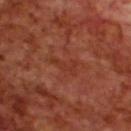Impression: No biopsy was performed on this lesion — it was imaged during a full skin examination and was not determined to be concerning. Image and clinical context: A male subject, about 70 years old. Approximately 3.5 mm at its widest. A 15 mm close-up tile from a total-body photography series done for melanoma screening. The total-body-photography lesion software estimated a lesion area of about 3.5 mm², an outline eccentricity of about 0.9 (0 = round, 1 = elongated), and a symmetry-axis asymmetry near 0.55. The analysis additionally found an average lesion color of about L≈34 a*≈27 b*≈30 (CIELAB), a lesion–skin lightness drop of about 5, and a normalized lesion–skin contrast near 5. Located on the upper back.